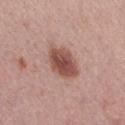  biopsy_status: not biopsied; imaged during a skin examination
  lighting: white-light
  image:
    source: total-body photography crop
    field_of_view_mm: 15
  lesion_size:
    long_diameter_mm_approx: 4.0
  patient:
    sex: female
    age_approx: 60
  site: right thigh